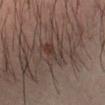Impression: The lesion was tiled from a total-body skin photograph and was not biopsied. Background: Captured under cross-polarized illumination. Cropped from a whole-body photographic skin survey; the tile spans about 15 mm. Measured at roughly 4 mm in maximum diameter. The lesion is located on the left forearm. Automated image analysis of the tile measured a footprint of about 8.5 mm², an outline eccentricity of about 0.5 (0 = round, 1 = elongated), and two-axis asymmetry of about 0.4. The analysis additionally found an average lesion color of about L≈32 a*≈13 b*≈18 (CIELAB), roughly 6 lightness units darker than nearby skin, and a normalized border contrast of about 6. The patient is a male aged 48 to 52.